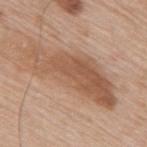notes = no biopsy performed (imaged during a skin exam)
body site = the upper back
acquisition = ~15 mm crop, total-body skin-cancer survey
image-analysis metrics = a footprint of about 32 mm², a shape eccentricity near 0.95, and two-axis asymmetry of about 0.35; a normalized lesion–skin contrast near 7.5; a border-irregularity rating of about 5.5/10, a color-variation rating of about 5.5/10, and a peripheral color-asymmetry measure near 2; a classifier nevus-likeness of about 30/100 and a detector confidence of about 100 out of 100 that the crop contains a lesion
patient = male, aged around 80
lighting = white-light illumination